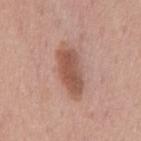Impression: Recorded during total-body skin imaging; not selected for excision or biopsy. Image and clinical context: This is a white-light tile. Measured at roughly 6.5 mm in maximum diameter. This image is a 15 mm lesion crop taken from a total-body photograph. The patient is a male about 55 years old. On the chest.